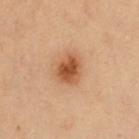biopsy_status: not biopsied; imaged during a skin examination
site: chest
image:
  source: total-body photography crop
  field_of_view_mm: 15
lesion_size:
  long_diameter_mm_approx: 3.5
patient:
  sex: female
  age_approx: 40
lighting: cross-polarized